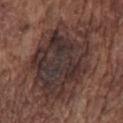location = the chest | acquisition = ~15 mm crop, total-body skin-cancer survey | lighting = white-light | subject = male, roughly 75 years of age | lesion diameter = ~12 mm (longest diameter).